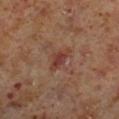follow-up: no biopsy performed (imaged during a skin exam)
subject: male, about 60 years old
imaging modality: total-body-photography crop, ~15 mm field of view
body site: the right lower leg
TBP lesion metrics: a footprint of about 5 mm² and two-axis asymmetry of about 0.3; a lesion-to-skin contrast of about 7 (normalized; higher = more distinct)